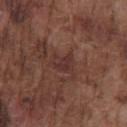Impression: The lesion was photographed on a routine skin check and not biopsied; there is no pathology result. Image and clinical context: The tile uses white-light illumination. About 2.5 mm across. A male patient in their mid- to late 70s. The lesion is on the chest. A region of skin cropped from a whole-body photographic capture, roughly 15 mm wide.